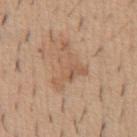site: front of the torso
automated_metrics:
  area_mm2_approx: 9.5
  eccentricity: 0.8
  shape_asymmetry: 0.75
  border_irregularity_0_10: 9.5
  color_variation_0_10: 3.0
  peripheral_color_asymmetry: 1.0
lighting: white-light
image:
  source: total-body photography crop
  field_of_view_mm: 15
lesion_size:
  long_diameter_mm_approx: 6.0
patient:
  sex: male
  age_approx: 40